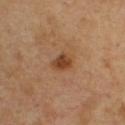Cropped from a whole-body photographic skin survey; the tile spans about 15 mm. The lesion is on the chest. A male patient aged around 50. The lesion's longest dimension is about 2.5 mm. Imaged with cross-polarized lighting.